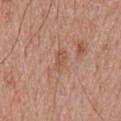Clinical impression:
The lesion was photographed on a routine skin check and not biopsied; there is no pathology result.
Acquisition and patient details:
A close-up tile cropped from a whole-body skin photograph, about 15 mm across. A male subject about 70 years old. Measured at roughly 2.5 mm in maximum diameter. On the head or neck. Automated tile analysis of the lesion measured a footprint of about 3.5 mm², an eccentricity of roughly 0.8, and a shape-asymmetry score of about 0.3 (0 = symmetric). The analysis additionally found a nevus-likeness score of about 0/100 and a lesion-detection confidence of about 100/100.